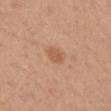Imaged during a routine full-body skin examination; the lesion was not biopsied and no histopathology is available.
A region of skin cropped from a whole-body photographic capture, roughly 15 mm wide.
An algorithmic analysis of the crop reported a border-irregularity index near 1.5/10, internal color variation of about 2 on a 0–10 scale, and peripheral color asymmetry of about 0.5. It also reported an automated nevus-likeness rating near 60 out of 100 and a detector confidence of about 100 out of 100 that the crop contains a lesion.
Measured at roughly 2.5 mm in maximum diameter.
The lesion is on the back.
Imaged with white-light lighting.
A female subject aged 33–37.